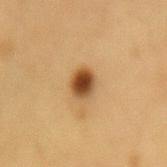The lesion was photographed on a routine skin check and not biopsied; there is no pathology result. On the lower back. A region of skin cropped from a whole-body photographic capture, roughly 15 mm wide. A male patient aged 53 to 57.